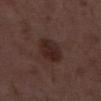Assessment:
Part of a total-body skin-imaging series; this lesion was reviewed on a skin check and was not flagged for biopsy.
Acquisition and patient details:
A male subject, in their 50s. A close-up tile cropped from a whole-body skin photograph, about 15 mm across. The tile uses white-light illumination. Longest diameter approximately 3.5 mm.